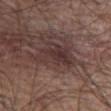Q: Is there a histopathology result?
A: total-body-photography surveillance lesion; no biopsy
Q: What is the lesion's diameter?
A: about 5.5 mm
Q: Who is the patient?
A: male, approximately 60 years of age
Q: Where on the body is the lesion?
A: the front of the torso
Q: How was this image acquired?
A: total-body-photography crop, ~15 mm field of view
Q: Illumination type?
A: white-light illumination